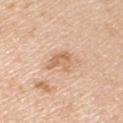Q: Is there a histopathology result?
A: imaged on a skin check; not biopsied
Q: Automated lesion metrics?
A: an area of roughly 6 mm², a shape eccentricity near 0.8, and a shape-asymmetry score of about 0.35 (0 = symmetric); an average lesion color of about L≈66 a*≈20 b*≈35 (CIELAB), roughly 9 lightness units darker than nearby skin, and a lesion-to-skin contrast of about 6.5 (normalized; higher = more distinct)
Q: What are the patient's age and sex?
A: male, approximately 50 years of age
Q: How large is the lesion?
A: ~4 mm (longest diameter)
Q: How was this image acquired?
A: total-body-photography crop, ~15 mm field of view
Q: Where on the body is the lesion?
A: the right upper arm